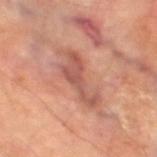The lesion is located on the leg. The tile uses cross-polarized illumination. Cropped from a whole-body photographic skin survey; the tile spans about 15 mm. Longest diameter approximately 6 mm. The patient is a male approximately 70 years of age. Automated tile analysis of the lesion measured a mean CIELAB color near L≈53 a*≈25 b*≈27, about 10 CIELAB-L* units darker than the surrounding skin, and a lesion-to-skin contrast of about 7 (normalized; higher = more distinct). And it measured border irregularity of about 8 on a 0–10 scale, internal color variation of about 2 on a 0–10 scale, and peripheral color asymmetry of about 0.5.